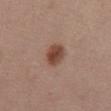Q: Was a biopsy performed?
A: catalogued during a skin exam; not biopsied
Q: Illumination type?
A: white-light illumination
Q: What is the imaging modality?
A: total-body-photography crop, ~15 mm field of view
Q: What is the lesion's diameter?
A: ~3 mm (longest diameter)
Q: What is the anatomic site?
A: the abdomen
Q: What are the patient's age and sex?
A: female, about 55 years old